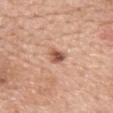notes — total-body-photography surveillance lesion; no biopsy
diameter — ≈2.5 mm
body site — the mid back
automated metrics — a mean CIELAB color near L≈55 a*≈24 b*≈30 and a normalized border contrast of about 9
image source — total-body-photography crop, ~15 mm field of view
patient — female, roughly 60 years of age